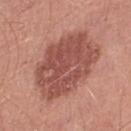Recorded during total-body skin imaging; not selected for excision or biopsy. On the left lower leg. Cropped from a whole-body photographic skin survey; the tile spans about 15 mm. A male patient aged 48–52.